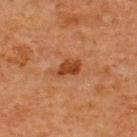Q: What kind of image is this?
A: total-body-photography crop, ~15 mm field of view
Q: Patient demographics?
A: male, aged approximately 65
Q: Lesion location?
A: the upper back